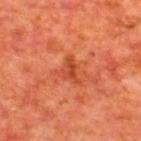Q: Where on the body is the lesion?
A: the back
Q: How large is the lesion?
A: ~3 mm (longest diameter)
Q: What kind of image is this?
A: ~15 mm tile from a whole-body skin photo
Q: Who is the patient?
A: male, in their mid-60s
Q: What lighting was used for the tile?
A: cross-polarized